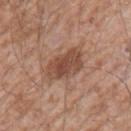No biopsy was performed on this lesion — it was imaged during a full skin examination and was not determined to be concerning. This is a white-light tile. The total-body-photography lesion software estimated a footprint of about 12 mm², an eccentricity of roughly 0.8, and a symmetry-axis asymmetry near 0.2. And it measured about 11 CIELAB-L* units darker than the surrounding skin and a lesion-to-skin contrast of about 8 (normalized; higher = more distinct). And it measured a border-irregularity index near 2/10, a within-lesion color-variation index near 4.5/10, and radial color variation of about 1.5. The analysis additionally found an automated nevus-likeness rating near 70 out of 100 and a detector confidence of about 100 out of 100 that the crop contains a lesion. Approximately 5 mm at its widest. A region of skin cropped from a whole-body photographic capture, roughly 15 mm wide. Located on the right upper arm. A male subject in their 60s.